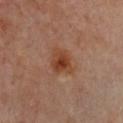No biopsy was performed on this lesion — it was imaged during a full skin examination and was not determined to be concerning.
The patient is a female roughly 60 years of age.
An algorithmic analysis of the crop reported an average lesion color of about L≈43 a*≈24 b*≈33 (CIELAB), a lesion–skin lightness drop of about 9, and a lesion-to-skin contrast of about 8.5 (normalized; higher = more distinct).
From the chest.
A 15 mm close-up extracted from a 3D total-body photography capture.
The recorded lesion diameter is about 3 mm.